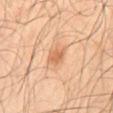Recorded during total-body skin imaging; not selected for excision or biopsy.
A male subject in their mid-60s.
This image is a 15 mm lesion crop taken from a total-body photograph.
Captured under cross-polarized illumination.
Measured at roughly 2.5 mm in maximum diameter.
The lesion-visualizer software estimated an area of roughly 4 mm² and a shape-asymmetry score of about 0.2 (0 = symmetric). The analysis additionally found a border-irregularity rating of about 2/10 and a peripheral color-asymmetry measure near 0.5. The software also gave a classifier nevus-likeness of about 80/100 and lesion-presence confidence of about 100/100.
On the mid back.